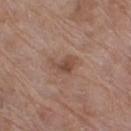Clinical impression: Part of a total-body skin-imaging series; this lesion was reviewed on a skin check and was not flagged for biopsy. Acquisition and patient details: A close-up tile cropped from a whole-body skin photograph, about 15 mm across. An algorithmic analysis of the crop reported a nevus-likeness score of about 5/100 and lesion-presence confidence of about 100/100. Longest diameter approximately 3 mm. Located on the right thigh. Captured under white-light illumination. A female patient aged approximately 70.